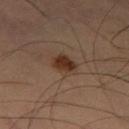Notes:
- image — total-body-photography crop, ~15 mm field of view
- anatomic site — the left thigh
- subject — male, aged 58–62
- lesion diameter — ~3.5 mm (longest diameter)
- lighting — cross-polarized
- TBP lesion metrics — a normalized lesion–skin contrast near 9.5